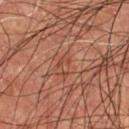Imaged during a routine full-body skin examination; the lesion was not biopsied and no histopathology is available. This is a cross-polarized tile. A region of skin cropped from a whole-body photographic capture, roughly 15 mm wide. On the chest. A male subject, about 55 years old. Approximately 3 mm at its widest.